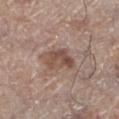Notes:
– follow-up · catalogued during a skin exam; not biopsied
– image-analysis metrics · a footprint of about 8 mm², an outline eccentricity of about 0.75 (0 = round, 1 = elongated), and a symmetry-axis asymmetry near 0.3; an automated nevus-likeness rating near 40 out of 100 and lesion-presence confidence of about 100/100
– patient · male, roughly 70 years of age
– lighting · white-light
– size · ≈4 mm
– body site · the left lower leg
– image · total-body-photography crop, ~15 mm field of view